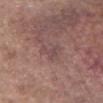The subject is a female approximately 65 years of age.
About 2.5 mm across.
From the left forearm.
Captured under white-light illumination.
This image is a 15 mm lesion crop taken from a total-body photograph.
Automated image analysis of the tile measured an average lesion color of about L≈46 a*≈19 b*≈18 (CIELAB), about 5 CIELAB-L* units darker than the surrounding skin, and a lesion-to-skin contrast of about 5 (normalized; higher = more distinct). It also reported a nevus-likeness score of about 0/100 and lesion-presence confidence of about 80/100.